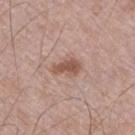{"biopsy_status": "not biopsied; imaged during a skin examination", "site": "right thigh", "lesion_size": {"long_diameter_mm_approx": 3.5}, "automated_metrics": {"area_mm2_approx": 5.5, "eccentricity": 0.85, "shape_asymmetry": 0.3, "border_irregularity_0_10": 3.0, "color_variation_0_10": 1.5, "peripheral_color_asymmetry": 0.5, "nevus_likeness_0_100": 80, "lesion_detection_confidence_0_100": 100}, "patient": {"sex": "male", "age_approx": 70}, "image": {"source": "total-body photography crop", "field_of_view_mm": 15}, "lighting": "white-light"}